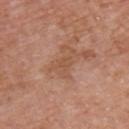| key | value |
|---|---|
| workup | no biopsy performed (imaged during a skin exam) |
| body site | the chest |
| image-analysis metrics | a lesion area of about 5 mm² and two-axis asymmetry of about 0.6; a nevus-likeness score of about 0/100 and lesion-presence confidence of about 100/100 |
| size | ~3.5 mm (longest diameter) |
| subject | male, approximately 70 years of age |
| acquisition | 15 mm crop, total-body photography |